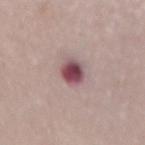The lesion is on the lower back. A male subject aged 73–77. A roughly 15 mm field-of-view crop from a total-body skin photograph. Imaged with white-light lighting.